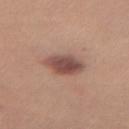Captured during whole-body skin photography for melanoma surveillance; the lesion was not biopsied.
A 15 mm close-up extracted from a 3D total-body photography capture.
Automated tile analysis of the lesion measured a shape eccentricity near 0.55 and a shape-asymmetry score of about 0.15 (0 = symmetric). It also reported a lesion color around L≈49 a*≈21 b*≈24 in CIELAB and roughly 13 lightness units darker than nearby skin. The analysis additionally found a border-irregularity rating of about 1.5/10. The analysis additionally found a classifier nevus-likeness of about 90/100.
A female subject, aged 23 to 27.
The lesion is on the right thigh.